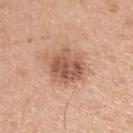Findings:
• subject · female, approximately 40 years of age
• image source · ~15 mm tile from a whole-body skin photo
• location · the left upper arm
• lighting · white-light illumination